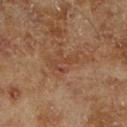follow-up: catalogued during a skin exam; not biopsied.